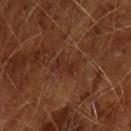Recorded during total-body skin imaging; not selected for excision or biopsy. A male patient, roughly 65 years of age. A roughly 15 mm field-of-view crop from a total-body skin photograph. An algorithmic analysis of the crop reported a symmetry-axis asymmetry near 0.4. And it measured a normalized border contrast of about 5.5. It also reported border irregularity of about 5 on a 0–10 scale, a within-lesion color-variation index near 2/10, and radial color variation of about 0.5. Approximately 3 mm at its widest.